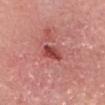No biopsy was performed on this lesion — it was imaged during a full skin examination and was not determined to be concerning.
A male patient aged 63–67.
This image is a 15 mm lesion crop taken from a total-body photograph.
Located on the head or neck.